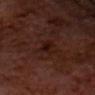biopsy status — no biopsy performed (imaged during a skin exam).